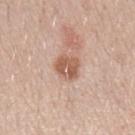This lesion was catalogued during total-body skin photography and was not selected for biopsy. About 3 mm across. Cropped from a total-body skin-imaging series; the visible field is about 15 mm. From the left forearm. The patient is a male aged 28 to 32.